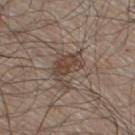follow-up=total-body-photography surveillance lesion; no biopsy
subject=male, aged 58–62
tile lighting=white-light
anatomic site=the leg
diameter=about 4.5 mm
imaging modality=~15 mm crop, total-body skin-cancer survey